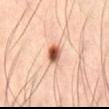The lesion was tiled from a total-body skin photograph and was not biopsied. Automated image analysis of the tile measured a footprint of about 4.5 mm², an outline eccentricity of about 0.7 (0 = round, 1 = elongated), and a shape-asymmetry score of about 0.2 (0 = symmetric). It also reported a border-irregularity index near 2/10 and a color-variation rating of about 8/10. Measured at roughly 2.5 mm in maximum diameter. This image is a 15 mm lesion crop taken from a total-body photograph. The subject is a male roughly 45 years of age. This is a cross-polarized tile. On the abdomen.